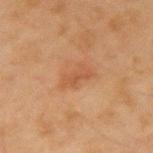Impression: No biopsy was performed on this lesion — it was imaged during a full skin examination and was not determined to be concerning. Image and clinical context: Cropped from a total-body skin-imaging series; the visible field is about 15 mm. The recorded lesion diameter is about 3 mm. From the left upper arm. A male subject, aged 43–47.